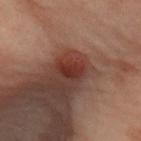| key | value |
|---|---|
| biopsy status | no biopsy performed (imaged during a skin exam) |
| acquisition | total-body-photography crop, ~15 mm field of view |
| location | the arm |
| subject | female, aged around 60 |